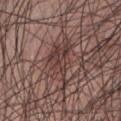Q: Was this lesion biopsied?
A: imaged on a skin check; not biopsied
Q: What is the imaging modality?
A: 15 mm crop, total-body photography
Q: Patient demographics?
A: male, in their 60s
Q: Where on the body is the lesion?
A: the abdomen